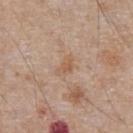notes = total-body-photography surveillance lesion; no biopsy | patient = male, aged 63 to 67 | location = the chest | image source = ~15 mm crop, total-body skin-cancer survey.